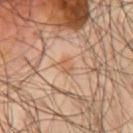A close-up tile cropped from a whole-body skin photograph, about 15 mm across.
The lesion is on the chest.
About 2 mm across.
A male patient aged around 55.
Automated image analysis of the tile measured a footprint of about 2.5 mm², an eccentricity of roughly 0.6, and a shape-asymmetry score of about 0.2 (0 = symmetric). It also reported a border-irregularity rating of about 1.5/10 and peripheral color asymmetry of about 0.5. The software also gave a nevus-likeness score of about 0/100 and a detector confidence of about 100 out of 100 that the crop contains a lesion.